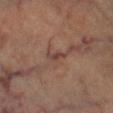Context:
The patient is a male aged 63 to 67. The lesion is on the left lower leg. A 15 mm crop from a total-body photograph taken for skin-cancer surveillance. This is a cross-polarized tile. About 2.5 mm across.